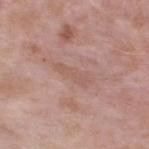No biopsy was performed on this lesion — it was imaged during a full skin examination and was not determined to be concerning. On the upper back. The patient is a male roughly 55 years of age. A roughly 15 mm field-of-view crop from a total-body skin photograph.